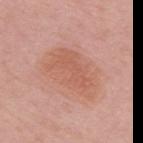This is a white-light tile.
A region of skin cropped from a whole-body photographic capture, roughly 15 mm wide.
From the upper back.
A female subject, approximately 60 years of age.